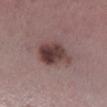Context: From the right lower leg. This is a white-light tile. Cropped from a whole-body photographic skin survey; the tile spans about 15 mm. About 5 mm across. A female patient in their mid- to late 40s.